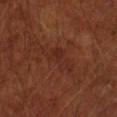No biopsy was performed on this lesion — it was imaged during a full skin examination and was not determined to be concerning.
Imaged with cross-polarized lighting.
A lesion tile, about 15 mm wide, cut from a 3D total-body photograph.
Located on the left forearm.
The recorded lesion diameter is about 5.5 mm.
The patient is a male about 65 years old.